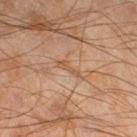  biopsy_status: not biopsied; imaged during a skin examination
  site: leg
  patient:
    sex: male
    age_approx: 45
  image:
    source: total-body photography crop
    field_of_view_mm: 15